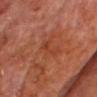This lesion was catalogued during total-body skin photography and was not selected for biopsy.
The lesion is located on the head or neck.
A male subject, aged around 65.
This image is a 15 mm lesion crop taken from a total-body photograph.